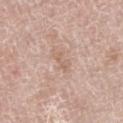notes = total-body-photography surveillance lesion; no biopsy | imaging modality = total-body-photography crop, ~15 mm field of view | location = the front of the torso | size = ~2.5 mm (longest diameter) | patient = female, aged around 60 | automated lesion analysis = a lesion area of about 3 mm², an eccentricity of roughly 0.8, and a symmetry-axis asymmetry near 0.35; an automated nevus-likeness rating near 0 out of 100 and lesion-presence confidence of about 100/100.